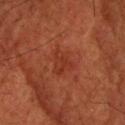This lesion was catalogued during total-body skin photography and was not selected for biopsy. The lesion is on the head or neck. The lesion's longest dimension is about 3.5 mm. Captured under cross-polarized illumination. A 15 mm crop from a total-body photograph taken for skin-cancer surveillance. A male subject, aged approximately 60.